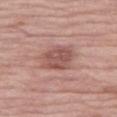Clinical impression:
The lesion was tiled from a total-body skin photograph and was not biopsied.
Acquisition and patient details:
This image is a 15 mm lesion crop taken from a total-body photograph. The lesion is located on the right thigh. The lesion-visualizer software estimated a footprint of about 10 mm², a shape eccentricity near 0.45, and a shape-asymmetry score of about 0.15 (0 = symmetric). And it measured about 10 CIELAB-L* units darker than the surrounding skin and a normalized border contrast of about 7. The patient is a female about 70 years old. The lesion's longest dimension is about 3.5 mm.